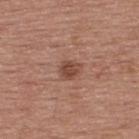biopsy status = catalogued during a skin exam; not biopsied
location = the upper back
size = ≈2.5 mm
lighting = white-light
patient = male, approximately 55 years of age
image = total-body-photography crop, ~15 mm field of view